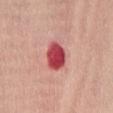Assessment:
The lesion was tiled from a total-body skin photograph and was not biopsied.
Context:
The lesion is on the chest. A 15 mm crop from a total-body photograph taken for skin-cancer surveillance. Captured under white-light illumination. Measured at roughly 3.5 mm in maximum diameter. A female patient, aged around 60.